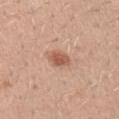- notes: no biopsy performed (imaged during a skin exam)
- site: the left upper arm
- patient: male, about 30 years old
- acquisition: total-body-photography crop, ~15 mm field of view
- tile lighting: white-light illumination